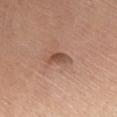Imaged during a routine full-body skin examination; the lesion was not biopsied and no histopathology is available. This is a white-light tile. From the upper back. Cropped from a total-body skin-imaging series; the visible field is about 15 mm. A male patient aged 63–67.